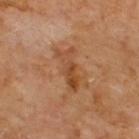workup: imaged on a skin check; not biopsied | patient: male, aged 68–72 | body site: the upper back | image source: 15 mm crop, total-body photography.